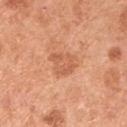Notes:
– notes: total-body-photography surveillance lesion; no biopsy
– subject: male, aged 53 to 57
– image-analysis metrics: an area of roughly 6.5 mm², an outline eccentricity of about 0.4 (0 = round, 1 = elongated), and two-axis asymmetry of about 0.35; an average lesion color of about L≈60 a*≈27 b*≈37 (CIELAB), a lesion–skin lightness drop of about 8, and a lesion-to-skin contrast of about 5 (normalized; higher = more distinct); a border-irregularity index near 3.5/10, a within-lesion color-variation index near 2.5/10, and radial color variation of about 1
– acquisition: 15 mm crop, total-body photography
– lesion diameter: about 3.5 mm
– body site: the left upper arm
– tile lighting: white-light illumination